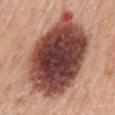notes = imaged on a skin check; not biopsied | anatomic site = the mid back | patient = male, roughly 70 years of age | image source = total-body-photography crop, ~15 mm field of view | lesion diameter = about 10.5 mm | illumination = white-light illumination.